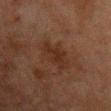biopsy status=total-body-photography surveillance lesion; no biopsy
acquisition=~15 mm crop, total-body skin-cancer survey
subject=male, aged 58 to 62
tile lighting=cross-polarized
size=≈3.5 mm
TBP lesion metrics=a lesion area of about 9 mm² and a shape eccentricity near 0.6; an average lesion color of about L≈22 a*≈16 b*≈22 (CIELAB), roughly 5 lightness units darker than nearby skin, and a normalized border contrast of about 6; a border-irregularity index near 3.5/10 and internal color variation of about 2 on a 0–10 scale
anatomic site=the chest